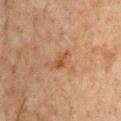Impression: The lesion was tiled from a total-body skin photograph and was not biopsied. Image and clinical context: The lesion is located on the chest. Captured under cross-polarized illumination. The subject is a male approximately 65 years of age. Cropped from a total-body skin-imaging series; the visible field is about 15 mm.